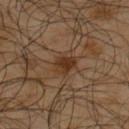Impression: The lesion was photographed on a routine skin check and not biopsied; there is no pathology result. Clinical summary: Automated image analysis of the tile measured an area of roughly 5.5 mm², an eccentricity of roughly 0.65, and a shape-asymmetry score of about 0.3 (0 = symmetric). The analysis additionally found a within-lesion color-variation index near 3.5/10 and radial color variation of about 1. The analysis additionally found a classifier nevus-likeness of about 70/100. On the upper back. A male patient, approximately 65 years of age. A roughly 15 mm field-of-view crop from a total-body skin photograph.